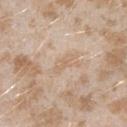Recorded during total-body skin imaging; not selected for excision or biopsy.
From the left upper arm.
A 15 mm close-up tile from a total-body photography series done for melanoma screening.
The tile uses white-light illumination.
About 3 mm across.
A female patient aged approximately 25.